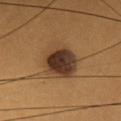A female patient, aged approximately 30. The lesion is located on the head or neck. Longest diameter approximately 5 mm. This is a cross-polarized tile. A roughly 15 mm field-of-view crop from a total-body skin photograph.